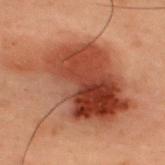biopsy_status: not biopsied; imaged during a skin examination
automated_metrics:
  cielab_L: 37
  cielab_a: 24
  cielab_b: 28
  vs_skin_darker_L: 13.0
  vs_skin_contrast_norm: 11.0
  border_irregularity_0_10: 5.0
  color_variation_0_10: 9.0
  peripheral_color_asymmetry: 3.0
  nevus_likeness_0_100: 55
image:
  source: total-body photography crop
  field_of_view_mm: 15
lesion_size:
  long_diameter_mm_approx: 11.5
site: upper back
patient:
  sex: male
  age_approx: 55